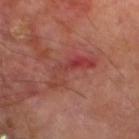  biopsy_status: not biopsied; imaged during a skin examination
  automated_metrics:
    border_irregularity_0_10: 8.5
    peripheral_color_asymmetry: 2.5
  lighting: cross-polarized
  patient:
    sex: male
    age_approx: 70
  image:
    source: total-body photography crop
    field_of_view_mm: 15
  lesion_size:
    long_diameter_mm_approx: 5.5
  site: left lower leg